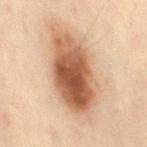* patient — female, in their mid- to late 40s
* image source — ~15 mm crop, total-body skin-cancer survey
* location — the right thigh